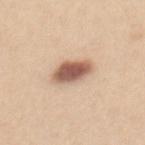Clinical impression: The lesion was tiled from a total-body skin photograph and was not biopsied. Acquisition and patient details: Captured under white-light illumination. Automated tile analysis of the lesion measured an area of roughly 9 mm², an outline eccentricity of about 0.75 (0 = round, 1 = elongated), and two-axis asymmetry of about 0.15. The software also gave a border-irregularity rating of about 1.5/10 and peripheral color asymmetry of about 1.5. A 15 mm close-up tile from a total-body photography series done for melanoma screening. A female patient, aged around 25. On the mid back. About 4 mm across.